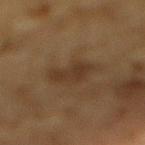No biopsy was performed on this lesion — it was imaged during a full skin examination and was not determined to be concerning.
A 15 mm crop from a total-body photograph taken for skin-cancer surveillance.
The lesion is on the back.
A male patient about 85 years old or older.